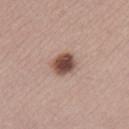Part of a total-body skin-imaging series; this lesion was reviewed on a skin check and was not flagged for biopsy. Automated tile analysis of the lesion measured a footprint of about 7 mm² and an outline eccentricity of about 0.6 (0 = round, 1 = elongated). The software also gave a nevus-likeness score of about 100/100 and a lesion-detection confidence of about 100/100. The lesion's longest dimension is about 3 mm. From the leg. A 15 mm crop from a total-body photograph taken for skin-cancer surveillance. This is a white-light tile. A female subject about 40 years old.